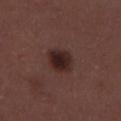- workup: imaged on a skin check; not biopsied
- image: ~15 mm crop, total-body skin-cancer survey
- patient: male, aged around 30
- location: the mid back
- illumination: white-light